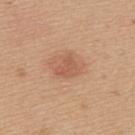Background:
A female patient aged 38 to 42. On the upper back. A 15 mm crop from a total-body photograph taken for skin-cancer surveillance. The lesion-visualizer software estimated an area of roughly 4 mm², an outline eccentricity of about 0.6 (0 = round, 1 = elongated), and two-axis asymmetry of about 0.4. And it measured a mean CIELAB color near L≈56 a*≈24 b*≈33, about 8 CIELAB-L* units darker than the surrounding skin, and a normalized lesion–skin contrast near 5. Captured under white-light illumination. The lesion's longest dimension is about 2.5 mm.